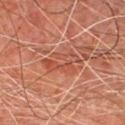Q: Was a biopsy performed?
A: total-body-photography surveillance lesion; no biopsy
Q: Automated lesion metrics?
A: about 7 CIELAB-L* units darker than the surrounding skin and a lesion-to-skin contrast of about 5.5 (normalized; higher = more distinct); a border-irregularity rating of about 8/10, a color-variation rating of about 7/10, and a peripheral color-asymmetry measure near 2; a classifier nevus-likeness of about 0/100 and a lesion-detection confidence of about 95/100
Q: Patient demographics?
A: male, aged approximately 65
Q: What kind of image is this?
A: total-body-photography crop, ~15 mm field of view
Q: Lesion size?
A: about 5 mm
Q: What is the anatomic site?
A: the chest